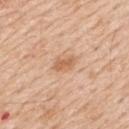Recorded during total-body skin imaging; not selected for excision or biopsy. Located on the upper back. Longest diameter approximately 3 mm. A male subject, about 60 years old. An algorithmic analysis of the crop reported a footprint of about 4 mm², a shape eccentricity near 0.85, and a symmetry-axis asymmetry near 0.25. And it measured a color-variation rating of about 1.5/10 and a peripheral color-asymmetry measure near 0.5. The analysis additionally found a nevus-likeness score of about 25/100 and lesion-presence confidence of about 100/100. A 15 mm close-up extracted from a 3D total-body photography capture.